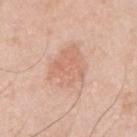The lesion's longest dimension is about 5.5 mm.
A 15 mm close-up extracted from a 3D total-body photography capture.
The subject is a male in their 70s.
The lesion is located on the right upper arm.